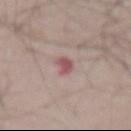* notes · catalogued during a skin exam; not biopsied
* location · the front of the torso
* TBP lesion metrics · a mean CIELAB color near L≈52 a*≈24 b*≈19, about 11 CIELAB-L* units darker than the surrounding skin, and a normalized lesion–skin contrast near 8
* size · ≈2.5 mm
* acquisition · total-body-photography crop, ~15 mm field of view
* illumination · white-light illumination
* patient · male, aged around 60